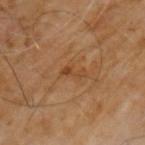biopsy status: no biopsy performed (imaged during a skin exam)
acquisition: 15 mm crop, total-body photography
site: the front of the torso
lesion size: about 3 mm
automated lesion analysis: a lesion area of about 2.5 mm², a shape eccentricity near 0.9, and a shape-asymmetry score of about 0.6 (0 = symmetric); border irregularity of about 7 on a 0–10 scale and a within-lesion color-variation index near 0/10; an automated nevus-likeness rating near 0 out of 100 and a lesion-detection confidence of about 100/100
subject: male, aged 58 to 62
lighting: cross-polarized